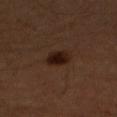Q: Was a biopsy performed?
A: no biopsy performed (imaged during a skin exam)
Q: Patient demographics?
A: male, aged 58–62
Q: What kind of image is this?
A: 15 mm crop, total-body photography
Q: What did automated image analysis measure?
A: an automated nevus-likeness rating near 100 out of 100 and a detector confidence of about 100 out of 100 that the crop contains a lesion
Q: What lighting was used for the tile?
A: cross-polarized
Q: Lesion location?
A: the arm